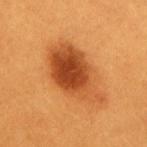biopsy status: catalogued during a skin exam; not biopsied | patient: female, aged approximately 30 | body site: the mid back | image-analysis metrics: a lesion area of about 24 mm², an outline eccentricity of about 0.85 (0 = round, 1 = elongated), and a shape-asymmetry score of about 0.2 (0 = symmetric); a lesion color around L≈45 a*≈29 b*≈41 in CIELAB, about 14 CIELAB-L* units darker than the surrounding skin, and a normalized border contrast of about 10; a color-variation rating of about 7/10 and a peripheral color-asymmetry measure near 2 | illumination: cross-polarized illumination | image source: ~15 mm crop, total-body skin-cancer survey.